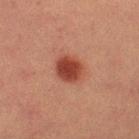Q: Was this lesion biopsied?
A: total-body-photography surveillance lesion; no biopsy
Q: How was this image acquired?
A: 15 mm crop, total-body photography
Q: Lesion size?
A: ~3.5 mm (longest diameter)
Q: What are the patient's age and sex?
A: female, roughly 55 years of age
Q: Lesion location?
A: the right lower leg
Q: Automated lesion metrics?
A: a lesion area of about 8.5 mm² and an eccentricity of roughly 0.5; a mean CIELAB color near L≈35 a*≈24 b*≈26 and about 11 CIELAB-L* units darker than the surrounding skin; internal color variation of about 3 on a 0–10 scale and radial color variation of about 1